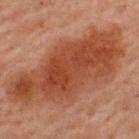{
  "biopsy_status": "not biopsied; imaged during a skin examination",
  "image": {
    "source": "total-body photography crop",
    "field_of_view_mm": 15
  },
  "patient": {
    "sex": "male",
    "age_approx": 60
  },
  "site": "upper back"
}